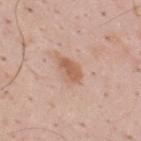| feature | finding |
|---|---|
| notes | catalogued during a skin exam; not biopsied |
| site | the back |
| subject | male, aged 33 to 37 |
| imaging modality | ~15 mm crop, total-body skin-cancer survey |
| diameter | ≈3.5 mm |